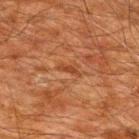No biopsy was performed on this lesion — it was imaged during a full skin examination and was not determined to be concerning. A male patient about 60 years old. Automated image analysis of the tile measured a lesion area of about 2.5 mm² and a symmetry-axis asymmetry near 0.3. And it measured a classifier nevus-likeness of about 0/100 and a lesion-detection confidence of about 50/100. The recorded lesion diameter is about 2.5 mm. On the upper back. A region of skin cropped from a whole-body photographic capture, roughly 15 mm wide. Imaged with cross-polarized lighting.